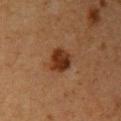follow-up — no biopsy performed (imaged during a skin exam); subject — female, aged around 40; imaging modality — 15 mm crop, total-body photography; automated lesion analysis — a lesion color around L≈27 a*≈20 b*≈28 in CIELAB and about 11 CIELAB-L* units darker than the surrounding skin; illumination — cross-polarized; site — the right upper arm.